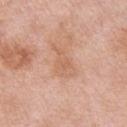Q: How large is the lesion?
A: about 4 mm
Q: What did automated image analysis measure?
A: a footprint of about 6.5 mm², an outline eccentricity of about 0.85 (0 = round, 1 = elongated), and a symmetry-axis asymmetry near 0.4; a mean CIELAB color near L≈62 a*≈22 b*≈32, roughly 6 lightness units darker than nearby skin, and a lesion-to-skin contrast of about 4.5 (normalized; higher = more distinct); border irregularity of about 4.5 on a 0–10 scale, a within-lesion color-variation index near 1.5/10, and a peripheral color-asymmetry measure near 0.5
Q: Lesion location?
A: the left upper arm
Q: What lighting was used for the tile?
A: white-light illumination
Q: Who is the patient?
A: male, in their mid- to late 50s
Q: What kind of image is this?
A: total-body-photography crop, ~15 mm field of view